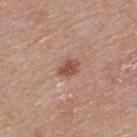notes — no biopsy performed (imaged during a skin exam)
patient — male, aged around 75
image source — 15 mm crop, total-body photography
diameter — ~2.5 mm (longest diameter)
TBP lesion metrics — a shape-asymmetry score of about 0.25 (0 = symmetric); a classifier nevus-likeness of about 85/100
body site — the back
tile lighting — white-light